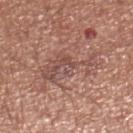This lesion was catalogued during total-body skin photography and was not selected for biopsy. A lesion tile, about 15 mm wide, cut from a 3D total-body photograph. A male patient, aged 68–72. Located on the right lower leg.